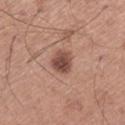This lesion was catalogued during total-body skin photography and was not selected for biopsy.
A 15 mm close-up extracted from a 3D total-body photography capture.
Captured under white-light illumination.
On the leg.
A male subject, aged 63–67.